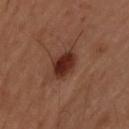biopsy status: no biopsy performed (imaged during a skin exam)
subject: male, aged around 35
tile lighting: cross-polarized
anatomic site: the upper back
image source: ~15 mm crop, total-body skin-cancer survey
size: ~3 mm (longest diameter)